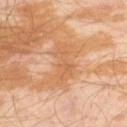Notes:
* workup — catalogued during a skin exam; not biopsied
* subject — male, about 30 years old
* lighting — cross-polarized illumination
* lesion diameter — ~5 mm (longest diameter)
* image source — 15 mm crop, total-body photography
* site — the left thigh